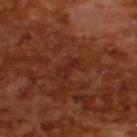Q: Was this lesion biopsied?
A: total-body-photography surveillance lesion; no biopsy
Q: What is the imaging modality?
A: ~15 mm tile from a whole-body skin photo
Q: What did automated image analysis measure?
A: an average lesion color of about L≈25 a*≈24 b*≈28 (CIELAB), about 5 CIELAB-L* units darker than the surrounding skin, and a normalized lesion–skin contrast near 5.5
Q: What is the lesion's diameter?
A: about 2.5 mm
Q: Illumination type?
A: cross-polarized illumination
Q: Patient demographics?
A: male, approximately 65 years of age